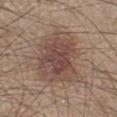{
  "biopsy_status": "not biopsied; imaged during a skin examination",
  "image": {
    "source": "total-body photography crop",
    "field_of_view_mm": 15
  },
  "lesion_size": {
    "long_diameter_mm_approx": 6.5
  },
  "lighting": "white-light",
  "site": "left lower leg",
  "patient": {
    "sex": "male",
    "age_approx": 45
  }
}